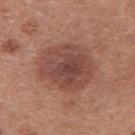biopsy status: imaged on a skin check; not biopsied | tile lighting: white-light illumination | image: total-body-photography crop, ~15 mm field of view | lesion diameter: ≈6 mm | subject: male, aged approximately 30 | site: the upper back.